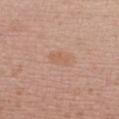Captured during whole-body skin photography for melanoma surveillance; the lesion was not biopsied. A 15 mm crop from a total-body photograph taken for skin-cancer surveillance. A female subject in their 60s. About 3 mm across. On the chest.